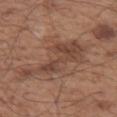notes: no biopsy performed (imaged during a skin exam)
imaging modality: total-body-photography crop, ~15 mm field of view
subject: male, approximately 55 years of age
lesion size: about 7.5 mm
anatomic site: the left upper arm
automated lesion analysis: an area of roughly 18 mm², an eccentricity of roughly 0.85, and two-axis asymmetry of about 0.55; a lesion color around L≈44 a*≈19 b*≈27 in CIELAB, roughly 8 lightness units darker than nearby skin, and a lesion-to-skin contrast of about 6.5 (normalized; higher = more distinct); a classifier nevus-likeness of about 0/100 and a lesion-detection confidence of about 95/100